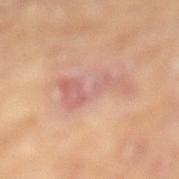Clinical impression: Part of a total-body skin-imaging series; this lesion was reviewed on a skin check and was not flagged for biopsy. Clinical summary: Approximately 6.5 mm at its widest. Automated tile analysis of the lesion measured a lesion-to-skin contrast of about 6 (normalized; higher = more distinct). And it measured a classifier nevus-likeness of about 0/100 and a lesion-detection confidence of about 100/100. This image is a 15 mm lesion crop taken from a total-body photograph. The lesion is located on the right lower leg. A female subject, in their mid- to late 70s. This is a cross-polarized tile.